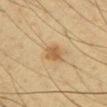Captured during whole-body skin photography for melanoma surveillance; the lesion was not biopsied. The patient is a male aged around 45. The lesion's longest dimension is about 2.5 mm. This is a cross-polarized tile. The lesion-visualizer software estimated a lesion area of about 4 mm², an outline eccentricity of about 0.6 (0 = round, 1 = elongated), and a shape-asymmetry score of about 0.25 (0 = symmetric). It also reported roughly 10 lightness units darker than nearby skin and a lesion-to-skin contrast of about 7.5 (normalized; higher = more distinct). Located on the chest. This image is a 15 mm lesion crop taken from a total-body photograph.